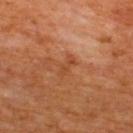biopsy status: total-body-photography surveillance lesion; no biopsy
subject: male, aged approximately 65
lighting: cross-polarized
diameter: ≈2.5 mm
acquisition: ~15 mm crop, total-body skin-cancer survey
location: the upper back